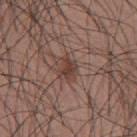Imaged during a routine full-body skin examination; the lesion was not biopsied and no histopathology is available. A male patient roughly 35 years of age. About 3 mm across. The lesion is located on the mid back. A 15 mm close-up tile from a total-body photography series done for melanoma screening. Captured under white-light illumination.